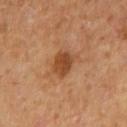{"biopsy_status": "not biopsied; imaged during a skin examination", "patient": {"sex": "male", "age_approx": 65}, "image": {"source": "total-body photography crop", "field_of_view_mm": 15}, "lesion_size": {"long_diameter_mm_approx": 3.5}, "site": "mid back", "lighting": "cross-polarized"}